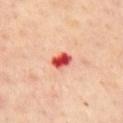Findings:
- follow-up — total-body-photography surveillance lesion; no biopsy
- body site — the chest
- TBP lesion metrics — an area of roughly 4 mm², an outline eccentricity of about 0.75 (0 = round, 1 = elongated), and a shape-asymmetry score of about 0.2 (0 = symmetric); a mean CIELAB color near L≈54 a*≈42 b*≈33; a nevus-likeness score of about 0/100 and a detector confidence of about 100 out of 100 that the crop contains a lesion
- image — ~15 mm crop, total-body skin-cancer survey
- patient — female, aged 43 to 47
- lesion diameter — about 2.5 mm